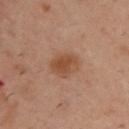{"biopsy_status": "not biopsied; imaged during a skin examination", "site": "upper back", "patient": {"sex": "male", "age_approx": 40}, "image": {"source": "total-body photography crop", "field_of_view_mm": 15}, "automated_metrics": {"nevus_likeness_0_100": 90, "lesion_detection_confidence_0_100": 100}, "lesion_size": {"long_diameter_mm_approx": 3.5}, "lighting": "cross-polarized"}